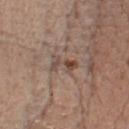notes — total-body-photography surveillance lesion; no biopsy
size — ~3 mm (longest diameter)
acquisition — ~15 mm tile from a whole-body skin photo
subject — male, aged 63 to 67
site — the chest
automated metrics — a mean CIELAB color near L≈47 a*≈16 b*≈24, about 9 CIELAB-L* units darker than the surrounding skin, and a normalized border contrast of about 7
lighting — white-light